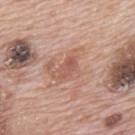Image and clinical context:
A lesion tile, about 15 mm wide, cut from a 3D total-body photograph. A male patient aged around 70. On the upper back. This is a white-light tile.